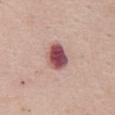No biopsy was performed on this lesion — it was imaged during a full skin examination and was not determined to be concerning.
This is a white-light tile.
The lesion is on the front of the torso.
Automated tile analysis of the lesion measured a lesion area of about 8 mm², a shape eccentricity near 0.6, and a shape-asymmetry score of about 0.2 (0 = symmetric). It also reported about 20 CIELAB-L* units darker than the surrounding skin and a normalized lesion–skin contrast near 13.5. The software also gave an automated nevus-likeness rating near 0 out of 100 and a lesion-detection confidence of about 100/100.
A female patient, roughly 65 years of age.
The recorded lesion diameter is about 3.5 mm.
Cropped from a whole-body photographic skin survey; the tile spans about 15 mm.